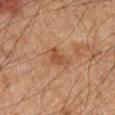The lesion was photographed on a routine skin check and not biopsied; there is no pathology result. The subject is a male aged 48–52. Approximately 2.5 mm at its widest. Imaged with cross-polarized lighting. A roughly 15 mm field-of-view crop from a total-body skin photograph. An algorithmic analysis of the crop reported an area of roughly 3.5 mm², an eccentricity of roughly 0.8, and a shape-asymmetry score of about 0.4 (0 = symmetric). The software also gave a border-irregularity index near 4/10 and peripheral color asymmetry of about 0. The analysis additionally found an automated nevus-likeness rating near 20 out of 100 and a detector confidence of about 100 out of 100 that the crop contains a lesion. The lesion is located on the left forearm.